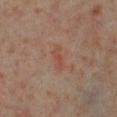Assessment: Imaged during a routine full-body skin examination; the lesion was not biopsied and no histopathology is available.